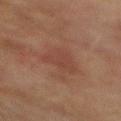follow-up: catalogued during a skin exam; not biopsied | imaging modality: ~15 mm tile from a whole-body skin photo | patient: male, aged approximately 75 | diameter: about 3.5 mm | illumination: cross-polarized illumination | automated metrics: an outline eccentricity of about 0.65 (0 = round, 1 = elongated) and two-axis asymmetry of about 0.5; an average lesion color of about L≈34 a*≈19 b*≈23 (CIELAB), a lesion–skin lightness drop of about 5, and a normalized lesion–skin contrast near 4.5; border irregularity of about 5 on a 0–10 scale, a within-lesion color-variation index near 1.5/10, and a peripheral color-asymmetry measure near 0.5 | body site: the back.